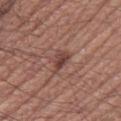Part of a total-body skin-imaging series; this lesion was reviewed on a skin check and was not flagged for biopsy. A male patient in their mid-50s. An algorithmic analysis of the crop reported an area of roughly 5 mm², a shape eccentricity near 0.7, and a symmetry-axis asymmetry near 0.4. And it measured a lesion color around L≈43 a*≈21 b*≈24 in CIELAB, roughly 9 lightness units darker than nearby skin, and a normalized border contrast of about 7.5. The software also gave a lesion-detection confidence of about 100/100. The lesion is located on the right thigh. Longest diameter approximately 3 mm. Cropped from a total-body skin-imaging series; the visible field is about 15 mm.